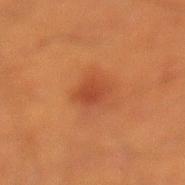Clinical impression:
Imaged during a routine full-body skin examination; the lesion was not biopsied and no histopathology is available.
Background:
Cropped from a total-body skin-imaging series; the visible field is about 15 mm. Longest diameter approximately 2.5 mm. An algorithmic analysis of the crop reported an average lesion color of about L≈38 a*≈27 b*≈32 (CIELAB), a lesion–skin lightness drop of about 7, and a lesion-to-skin contrast of about 6.5 (normalized; higher = more distinct). It also reported an automated nevus-likeness rating near 80 out of 100 and lesion-presence confidence of about 100/100. The tile uses cross-polarized illumination. The lesion is on the left lower leg. The patient is a male approximately 40 years of age.